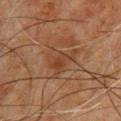The lesion was photographed on a routine skin check and not biopsied; there is no pathology result. Longest diameter approximately 4 mm. A male subject aged 58 to 62. The tile uses cross-polarized illumination. A close-up tile cropped from a whole-body skin photograph, about 15 mm across. The total-body-photography lesion software estimated a footprint of about 7.5 mm², an eccentricity of roughly 0.65, and a shape-asymmetry score of about 0.5 (0 = symmetric). It also reported an average lesion color of about L≈34 a*≈18 b*≈28 (CIELAB) and a lesion–skin lightness drop of about 6. It also reported border irregularity of about 6 on a 0–10 scale and a color-variation rating of about 4/10. And it measured an automated nevus-likeness rating near 0 out of 100 and lesion-presence confidence of about 95/100. Located on the front of the torso.